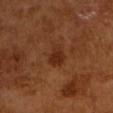Measured at roughly 2.5 mm in maximum diameter.
This is a cross-polarized tile.
Cropped from a whole-body photographic skin survey; the tile spans about 15 mm.
The subject is a male roughly 65 years of age.